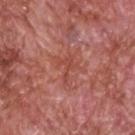Assessment: The lesion was photographed on a routine skin check and not biopsied; there is no pathology result. Clinical summary: A male patient aged around 60. Automated image analysis of the tile measured a shape eccentricity near 0.8. The software also gave a lesion color around L≈48 a*≈29 b*≈29 in CIELAB, about 7 CIELAB-L* units darker than the surrounding skin, and a normalized border contrast of about 5.5. A 15 mm close-up extracted from a 3D total-body photography capture. The lesion is on the head or neck. About 3 mm across. Captured under white-light illumination.